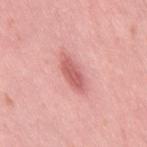  biopsy_status: not biopsied; imaged during a skin examination
  image:
    source: total-body photography crop
    field_of_view_mm: 15
  site: back
  lesion_size:
    long_diameter_mm_approx: 5.0
  patient:
    sex: female
    age_approx: 15